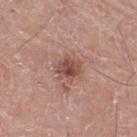The lesion was tiled from a total-body skin photograph and was not biopsied.
Measured at roughly 3 mm in maximum diameter.
The patient is a male aged 73 to 77.
Located on the right thigh.
A roughly 15 mm field-of-view crop from a total-body skin photograph.